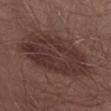* notes — no biopsy performed (imaged during a skin exam)
* site — the abdomen
* subject — male, in their mid-50s
* image — total-body-photography crop, ~15 mm field of view
* diameter — ~10 mm (longest diameter)
* illumination — white-light